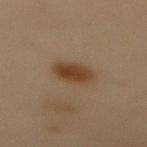Captured under cross-polarized illumination.
Measured at roughly 4 mm in maximum diameter.
On the mid back.
A 15 mm crop from a total-body photograph taken for skin-cancer surveillance.
Automated image analysis of the tile measured a border-irregularity rating of about 2/10 and a color-variation rating of about 3/10. The analysis additionally found an automated nevus-likeness rating near 100 out of 100 and lesion-presence confidence of about 100/100.
The patient is a female roughly 50 years of age.